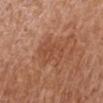{
  "biopsy_status": "not biopsied; imaged during a skin examination",
  "site": "left arm",
  "lighting": "white-light",
  "patient": {
    "sex": "female",
    "age_approx": 65
  },
  "lesion_size": {
    "long_diameter_mm_approx": 4.5
  },
  "image": {
    "source": "total-body photography crop",
    "field_of_view_mm": 15
  }
}